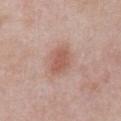Part of a total-body skin-imaging series; this lesion was reviewed on a skin check and was not flagged for biopsy.
Cropped from a total-body skin-imaging series; the visible field is about 15 mm.
The recorded lesion diameter is about 3.5 mm.
The patient is a male aged approximately 55.
The tile uses white-light illumination.
The lesion is on the abdomen.
The lesion-visualizer software estimated an area of roughly 7.5 mm², an eccentricity of roughly 0.7, and a shape-asymmetry score of about 0.2 (0 = symmetric). The analysis additionally found an automated nevus-likeness rating near 90 out of 100 and a lesion-detection confidence of about 100/100.